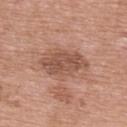follow-up=catalogued during a skin exam; not biopsied | subject=female, about 70 years old | TBP lesion metrics=an area of roughly 13 mm²; a border-irregularity index near 2.5/10 and a peripheral color-asymmetry measure near 1; a nevus-likeness score of about 0/100 and lesion-presence confidence of about 100/100 | image=total-body-photography crop, ~15 mm field of view | tile lighting=white-light illumination | anatomic site=the back.